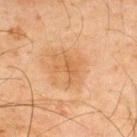Assessment: Part of a total-body skin-imaging series; this lesion was reviewed on a skin check and was not flagged for biopsy. Background: A male patient aged around 45. The lesion is located on the back. Cropped from a total-body skin-imaging series; the visible field is about 15 mm. Approximately 2.5 mm at its widest.